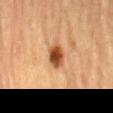| field | value |
|---|---|
| notes | no biopsy performed (imaged during a skin exam) |
| patient | male, aged 83 or older |
| automated metrics | a lesion color around L≈44 a*≈23 b*≈35 in CIELAB, a lesion–skin lightness drop of about 16, and a lesion-to-skin contrast of about 11.5 (normalized; higher = more distinct); border irregularity of about 2 on a 0–10 scale and peripheral color asymmetry of about 2 |
| size | ~3.5 mm (longest diameter) |
| acquisition | ~15 mm tile from a whole-body skin photo |
| location | the mid back |